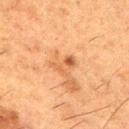Assessment:
This lesion was catalogued during total-body skin photography and was not selected for biopsy.
Context:
Longest diameter approximately 3 mm. A region of skin cropped from a whole-body photographic capture, roughly 15 mm wide. A male subject approximately 50 years of age. The lesion is located on the arm. This is a cross-polarized tile.